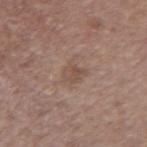Captured during whole-body skin photography for melanoma surveillance; the lesion was not biopsied.